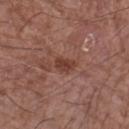The lesion was photographed on a routine skin check and not biopsied; there is no pathology result.
Approximately 2.5 mm at its widest.
A region of skin cropped from a whole-body photographic capture, roughly 15 mm wide.
A male patient, aged 68 to 72.
An algorithmic analysis of the crop reported an area of roughly 3.5 mm², an eccentricity of roughly 0.75, and a symmetry-axis asymmetry near 0.25.
The lesion is located on the left forearm.
Captured under white-light illumination.